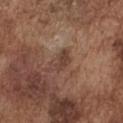Recorded during total-body skin imaging; not selected for excision or biopsy.
The recorded lesion diameter is about 3 mm.
A male subject, about 75 years old.
Captured under white-light illumination.
The lesion-visualizer software estimated a footprint of about 4 mm², a shape eccentricity near 0.85, and a symmetry-axis asymmetry near 0.45. It also reported a border-irregularity index near 5/10.
A region of skin cropped from a whole-body photographic capture, roughly 15 mm wide.
From the chest.